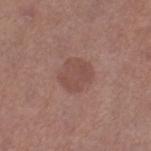Impression: Captured during whole-body skin photography for melanoma surveillance; the lesion was not biopsied. Image and clinical context: Imaged with white-light lighting. A female subject, aged 48–52. This image is a 15 mm lesion crop taken from a total-body photograph. Located on the left thigh. The recorded lesion diameter is about 3.5 mm. An algorithmic analysis of the crop reported a footprint of about 9.5 mm², an eccentricity of roughly 0.45, and a symmetry-axis asymmetry near 0.2.